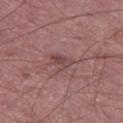Assessment: This lesion was catalogued during total-body skin photography and was not selected for biopsy. Context: Cropped from a whole-body photographic skin survey; the tile spans about 15 mm. The lesion is located on the left thigh. Imaged with white-light lighting. Approximately 2.5 mm at its widest. The subject is a male aged 58–62.